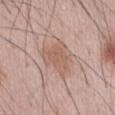follow-up: no biopsy performed (imaged during a skin exam)
tile lighting: white-light illumination
patient: male, approximately 60 years of age
diameter: ~5 mm (longest diameter)
location: the abdomen
acquisition: 15 mm crop, total-body photography